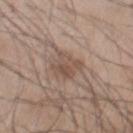Assessment:
Imaged during a routine full-body skin examination; the lesion was not biopsied and no histopathology is available.
Acquisition and patient details:
The lesion-visualizer software estimated an area of roughly 8 mm², an outline eccentricity of about 0.75 (0 = round, 1 = elongated), and a symmetry-axis asymmetry near 0.3. The analysis additionally found a border-irregularity rating of about 3.5/10, internal color variation of about 4 on a 0–10 scale, and radial color variation of about 1.5. Measured at roughly 4 mm in maximum diameter. A male subject, aged around 55. The tile uses white-light illumination. The lesion is on the front of the torso. This image is a 15 mm lesion crop taken from a total-body photograph.